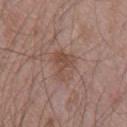workup: catalogued during a skin exam; not biopsied | location: the chest | subject: male, aged 68 to 72 | automated metrics: a footprint of about 6 mm² and an outline eccentricity of about 0.45 (0 = round, 1 = elongated); an average lesion color of about L≈48 a*≈19 b*≈25 (CIELAB) and a normalized lesion–skin contrast near 6; a nevus-likeness score of about 0/100 | tile lighting: white-light illumination | imaging modality: 15 mm crop, total-body photography.